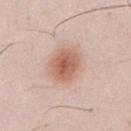Clinical impression:
The lesion was photographed on a routine skin check and not biopsied; there is no pathology result.
Acquisition and patient details:
The lesion is located on the abdomen. Cropped from a total-body skin-imaging series; the visible field is about 15 mm. The total-body-photography lesion software estimated a mean CIELAB color near L≈61 a*≈22 b*≈29 and a normalized border contrast of about 8. A male patient, approximately 35 years of age. Captured under white-light illumination.